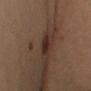| field | value |
|---|---|
| patient | female, aged approximately 35 |
| TBP lesion metrics | a border-irregularity index near 2.5/10, a color-variation rating of about 2.5/10, and radial color variation of about 1 |
| diameter | about 3 mm |
| image | ~15 mm crop, total-body skin-cancer survey |
| anatomic site | the right upper arm |
| illumination | cross-polarized illumination |